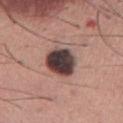follow-up = total-body-photography surveillance lesion; no biopsy | illumination = white-light | image = ~15 mm crop, total-body skin-cancer survey | size = about 4 mm | patient = male, aged 43–47 | location = the front of the torso.